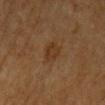Captured during whole-body skin photography for melanoma surveillance; the lesion was not biopsied.
On the upper back.
A 15 mm close-up tile from a total-body photography series done for melanoma screening.
Automated image analysis of the tile measured an area of roughly 4.5 mm² and a shape eccentricity near 0.5.
A female patient, about 55 years old.
Captured under cross-polarized illumination.
The lesion's longest dimension is about 2.5 mm.